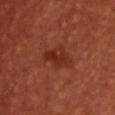The lesion was tiled from a total-body skin photograph and was not biopsied.
Cropped from a whole-body photographic skin survey; the tile spans about 15 mm.
This is a cross-polarized tile.
An algorithmic analysis of the crop reported a footprint of about 7 mm², a shape eccentricity near 0.75, and two-axis asymmetry of about 0.25. It also reported an average lesion color of about L≈30 a*≈29 b*≈32 (CIELAB) and a lesion–skin lightness drop of about 7. It also reported an automated nevus-likeness rating near 35 out of 100 and a detector confidence of about 100 out of 100 that the crop contains a lesion.
The subject is a female roughly 45 years of age.
The lesion is on the front of the torso.